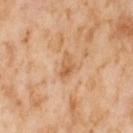<tbp_lesion>
<biopsy_status>not biopsied; imaged during a skin examination</biopsy_status>
<patient>
  <sex>female</sex>
  <age_approx>55</age_approx>
</patient>
<lighting>cross-polarized</lighting>
<lesion_size>
  <long_diameter_mm_approx>3.0</long_diameter_mm_approx>
</lesion_size>
<site>right thigh</site>
<image>
  <source>total-body photography crop</source>
  <field_of_view_mm>15</field_of_view_mm>
</image>
<automated_metrics>
  <eccentricity>0.8</eccentricity>
  <shape_asymmetry>0.4</shape_asymmetry>
  <vs_skin_darker_L>9.0</vs_skin_darker_L>
  <vs_skin_contrast_norm>6.0</vs_skin_contrast_norm>
</automated_metrics>
</tbp_lesion>